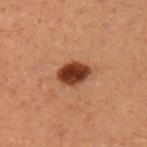biopsy status = imaged on a skin check; not biopsied